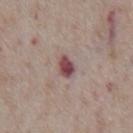{
  "biopsy_status": "not biopsied; imaged during a skin examination",
  "site": "chest",
  "image": {
    "source": "total-body photography crop",
    "field_of_view_mm": 15
  },
  "lesion_size": {
    "long_diameter_mm_approx": 2.5
  },
  "lighting": "white-light",
  "automated_metrics": {
    "border_irregularity_0_10": 2.0,
    "color_variation_0_10": 4.0,
    "peripheral_color_asymmetry": 1.5,
    "nevus_likeness_0_100": 5,
    "lesion_detection_confidence_0_100": 100
  },
  "patient": {
    "sex": "male",
    "age_approx": 75
  }
}